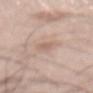biopsy status = no biopsy performed (imaged during a skin exam) | subject = male, aged around 55 | site = the back | imaging modality = total-body-photography crop, ~15 mm field of view | lesion diameter = ≈4.5 mm.